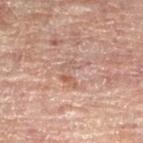Captured during whole-body skin photography for melanoma surveillance; the lesion was not biopsied. Cropped from a total-body skin-imaging series; the visible field is about 15 mm. Imaged with cross-polarized lighting. A female subject, in their 80s. An algorithmic analysis of the crop reported a mean CIELAB color near L≈53 a*≈18 b*≈25 and roughly 6 lightness units darker than nearby skin. The software also gave border irregularity of about 8.5 on a 0–10 scale, internal color variation of about 1.5 on a 0–10 scale, and radial color variation of about 0. It also reported a classifier nevus-likeness of about 0/100 and a detector confidence of about 100 out of 100 that the crop contains a lesion. Located on the right leg. Approximately 3.5 mm at its widest.